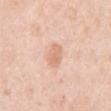biopsy status — no biopsy performed (imaged during a skin exam)
patient — female, in their mid- to late 60s
acquisition — 15 mm crop, total-body photography
anatomic site — the right upper arm
illumination — white-light illumination
lesion size — ~3 mm (longest diameter)